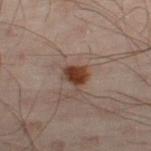The lesion was tiled from a total-body skin photograph and was not biopsied.
On the left leg.
A male subject aged around 50.
A 15 mm close-up extracted from a 3D total-body photography capture.
The recorded lesion diameter is about 3 mm.
Imaged with cross-polarized lighting.
Automated tile analysis of the lesion measured a classifier nevus-likeness of about 95/100 and a lesion-detection confidence of about 100/100.